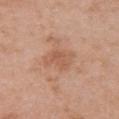| feature | finding |
|---|---|
| workup | imaged on a skin check; not biopsied |
| diameter | ≈3 mm |
| patient | female, roughly 40 years of age |
| body site | the left upper arm |
| image | 15 mm crop, total-body photography |
| illumination | white-light illumination |
| automated metrics | a footprint of about 6.5 mm², an eccentricity of roughly 0.65, and a shape-asymmetry score of about 0.35 (0 = symmetric); an average lesion color of about L≈57 a*≈22 b*≈32 (CIELAB), about 7 CIELAB-L* units darker than the surrounding skin, and a normalized border contrast of about 5 |